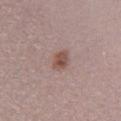Q: Was this lesion biopsied?
A: catalogued during a skin exam; not biopsied